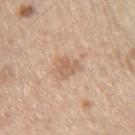The lesion-visualizer software estimated a border-irregularity index near 3.5/10, internal color variation of about 2.5 on a 0–10 scale, and radial color variation of about 1.
The subject is a male aged approximately 70.
A 15 mm crop from a total-body photograph taken for skin-cancer surveillance.
The tile uses white-light illumination.
On the right thigh.